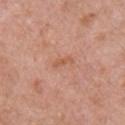Imaged during a routine full-body skin examination; the lesion was not biopsied and no histopathology is available. Approximately 2.5 mm at its widest. The lesion is located on the right upper arm. Automated image analysis of the tile measured an area of roughly 2.5 mm², a shape eccentricity near 0.95, and a shape-asymmetry score of about 0.35 (0 = symmetric). The software also gave an average lesion color of about L≈57 a*≈24 b*≈33 (CIELAB), roughly 7 lightness units darker than nearby skin, and a normalized border contrast of about 5.5. The software also gave a nevus-likeness score of about 0/100 and a detector confidence of about 100 out of 100 that the crop contains a lesion. This is a white-light tile. The subject is a female aged around 60. A region of skin cropped from a whole-body photographic capture, roughly 15 mm wide.